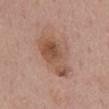This lesion was catalogued during total-body skin photography and was not selected for biopsy.
The tile uses white-light illumination.
Located on the mid back.
The total-body-photography lesion software estimated an average lesion color of about L≈53 a*≈20 b*≈29 (CIELAB), a lesion–skin lightness drop of about 11, and a lesion-to-skin contrast of about 8 (normalized; higher = more distinct). The software also gave a border-irregularity rating of about 4/10, a color-variation rating of about 7/10, and peripheral color asymmetry of about 2.
The subject is a male approximately 60 years of age.
A 15 mm close-up tile from a total-body photography series done for melanoma screening.